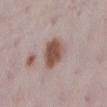• workup · imaged on a skin check; not biopsied
• body site · the left lower leg
• image source · ~15 mm tile from a whole-body skin photo
• subject · female, aged 28 to 32